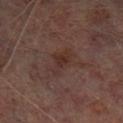No biopsy was performed on this lesion — it was imaged during a full skin examination and was not determined to be concerning. This is a cross-polarized tile. The lesion's longest dimension is about 4 mm. From the left lower leg. The subject is a male aged approximately 65. A region of skin cropped from a whole-body photographic capture, roughly 15 mm wide.